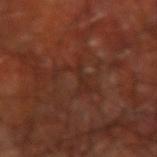workup: total-body-photography surveillance lesion; no biopsy
image-analysis metrics: a mean CIELAB color near L≈27 a*≈22 b*≈25 and about 4 CIELAB-L* units darker than the surrounding skin; a classifier nevus-likeness of about 0/100 and a detector confidence of about 80 out of 100 that the crop contains a lesion
lighting: cross-polarized
patient: male, about 60 years old
site: the arm
image: ~15 mm tile from a whole-body skin photo